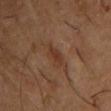  biopsy_status: not biopsied; imaged during a skin examination
  lesion_size:
    long_diameter_mm_approx: 3.0
  site: front of the torso
  automated_metrics:
    area_mm2_approx: 4.0
    eccentricity: 0.85
    shape_asymmetry: 0.3
    vs_skin_darker_L: 6.0
    vs_skin_contrast_norm: 6.0
    nevus_likeness_0_100: 0
    lesion_detection_confidence_0_100: 100
  patient:
    sex: male
    age_approx: 55
  lighting: cross-polarized
  image:
    source: total-body photography crop
    field_of_view_mm: 15